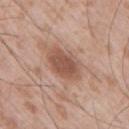site: right upper arm
patient:
  sex: male
  age_approx: 50
lesion_size:
  long_diameter_mm_approx: 4.5
image:
  source: total-body photography crop
  field_of_view_mm: 15
lighting: white-light